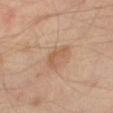The lesion is located on the right thigh.
A subject aged around 55.
The tile uses cross-polarized illumination.
Cropped from a whole-body photographic skin survey; the tile spans about 15 mm.
About 3.5 mm across.